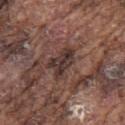Findings:
- TBP lesion metrics: a nevus-likeness score of about 0/100
- anatomic site: the left upper arm
- imaging modality: ~15 mm tile from a whole-body skin photo
- diameter: ≈4 mm
- subject: male, in their mid- to late 70s